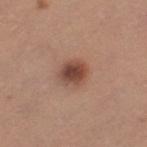Recorded during total-body skin imaging; not selected for excision or biopsy.
A female patient, aged 33–37.
A region of skin cropped from a whole-body photographic capture, roughly 15 mm wide.
From the left thigh.
Captured under white-light illumination.
About 3 mm across.